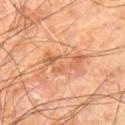{"biopsy_status": "not biopsied; imaged during a skin examination", "patient": {"sex": "male", "age_approx": 60}, "automated_metrics": {"cielab_L": 60, "cielab_a": 25, "cielab_b": 37, "vs_skin_darker_L": 9.0, "vs_skin_contrast_norm": 6.0, "border_irregularity_0_10": 5.5, "color_variation_0_10": 6.0, "peripheral_color_asymmetry": 2.0}, "site": "right leg", "lesion_size": {"long_diameter_mm_approx": 5.5}, "image": {"source": "total-body photography crop", "field_of_view_mm": 15}, "lighting": "cross-polarized"}